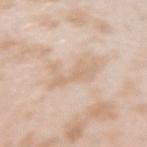Acquisition and patient details:
Measured at roughly 5 mm in maximum diameter. This image is a 15 mm lesion crop taken from a total-body photograph. Captured under white-light illumination. An algorithmic analysis of the crop reported a lesion area of about 9 mm², an eccentricity of roughly 0.85, and a shape-asymmetry score of about 0.5 (0 = symmetric). And it measured a mean CIELAB color near L≈69 a*≈15 b*≈30, about 6 CIELAB-L* units darker than the surrounding skin, and a lesion-to-skin contrast of about 4.5 (normalized; higher = more distinct). And it measured a classifier nevus-likeness of about 0/100. The lesion is located on the left upper arm. The subject is a female in their mid-20s.